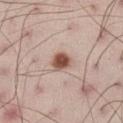| key | value |
|---|---|
| follow-up | catalogued during a skin exam; not biopsied |
| illumination | white-light |
| anatomic site | the right thigh |
| image source | total-body-photography crop, ~15 mm field of view |
| lesion diameter | about 2.5 mm |
| subject | male, approximately 30 years of age |